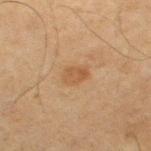{
  "image": {
    "source": "total-body photography crop",
    "field_of_view_mm": 15
  },
  "site": "left thigh",
  "patient": {
    "sex": "male",
    "age_approx": 65
  }
}